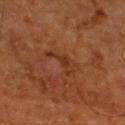The lesion was photographed on a routine skin check and not biopsied; there is no pathology result.
On the upper back.
Cropped from a whole-body photographic skin survey; the tile spans about 15 mm.
The tile uses cross-polarized illumination.
The patient is a male aged around 55.